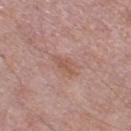workup = no biopsy performed (imaged during a skin exam); patient = male, roughly 65 years of age; site = the leg; acquisition = 15 mm crop, total-body photography.